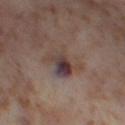Impression: No biopsy was performed on this lesion — it was imaged during a full skin examination and was not determined to be concerning. Background: A 15 mm crop from a total-body photograph taken for skin-cancer surveillance. The lesion is on the right thigh. The subject is a female aged around 55.